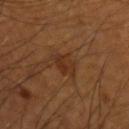Case summary:
- workup — total-body-photography surveillance lesion; no biopsy
- site — the left forearm
- diameter — ~3 mm (longest diameter)
- acquisition — ~15 mm crop, total-body skin-cancer survey
- tile lighting — cross-polarized
- patient — male, aged 53–57